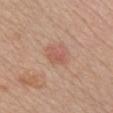Q: Was a biopsy performed?
A: total-body-photography surveillance lesion; no biopsy